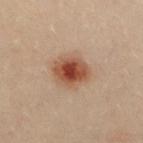Case summary:
* follow-up: no biopsy performed (imaged during a skin exam)
* lighting: cross-polarized illumination
* TBP lesion metrics: border irregularity of about 1 on a 0–10 scale, internal color variation of about 5 on a 0–10 scale, and a peripheral color-asymmetry measure near 1; an automated nevus-likeness rating near 100 out of 100 and lesion-presence confidence of about 100/100
* diameter: about 4 mm
* image source: total-body-photography crop, ~15 mm field of view
* patient: female, about 30 years old
* location: the chest